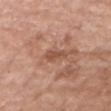Clinical impression: The lesion was photographed on a routine skin check and not biopsied; there is no pathology result. Acquisition and patient details: The patient is a male roughly 75 years of age. A lesion tile, about 15 mm wide, cut from a 3D total-body photograph. Located on the head or neck.